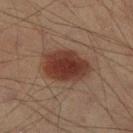This lesion was catalogued during total-body skin photography and was not selected for biopsy.
About 6 mm across.
Automated image analysis of the tile measured a border-irregularity index near 2/10. The analysis additionally found a classifier nevus-likeness of about 100/100.
A roughly 15 mm field-of-view crop from a total-body skin photograph.
A male subject, aged around 40.
The tile uses cross-polarized illumination.
From the right lower leg.